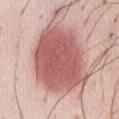<lesion>
  <site>abdomen</site>
  <patient>
    <sex>female</sex>
    <age_approx>50</age_approx>
  </patient>
  <image>
    <source>total-body photography crop</source>
    <field_of_view_mm>15</field_of_view_mm>
  </image>
</lesion>